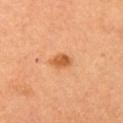<record>
  <biopsy_status>not biopsied; imaged during a skin examination</biopsy_status>
  <automated_metrics>
    <peripheral_color_asymmetry>1.0</peripheral_color_asymmetry>
    <nevus_likeness_0_100>85</nevus_likeness_0_100>
    <lesion_detection_confidence_0_100>100</lesion_detection_confidence_0_100>
  </automated_metrics>
  <site>left upper arm</site>
  <lesion_size>
    <long_diameter_mm_approx>2.5</long_diameter_mm_approx>
  </lesion_size>
  <patient>
    <sex>female</sex>
    <age_approx>35</age_approx>
  </patient>
  <image>
    <source>total-body photography crop</source>
    <field_of_view_mm>15</field_of_view_mm>
  </image>
  <lighting>cross-polarized</lighting>
</record>